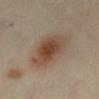| feature | finding |
|---|---|
| notes | total-body-photography surveillance lesion; no biopsy |
| diameter | ~6.5 mm (longest diameter) |
| image-analysis metrics | a mean CIELAB color near L≈47 a*≈17 b*≈28, a lesion–skin lightness drop of about 11, and a normalized border contrast of about 9; border irregularity of about 2.5 on a 0–10 scale, a within-lesion color-variation index near 5.5/10, and peripheral color asymmetry of about 1.5 |
| patient | female, roughly 55 years of age |
| acquisition | ~15 mm tile from a whole-body skin photo |
| body site | the abdomen |